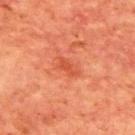Notes:
• notes: catalogued during a skin exam; not biopsied
• patient: male, approximately 65 years of age
• lesion size: about 2.5 mm
• anatomic site: the upper back
• image: 15 mm crop, total-body photography